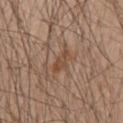Assessment:
Captured during whole-body skin photography for melanoma surveillance; the lesion was not biopsied.
Image and clinical context:
The recorded lesion diameter is about 3 mm. The lesion is on the mid back. A male subject aged approximately 60. An algorithmic analysis of the crop reported a symmetry-axis asymmetry near 0.25. This is a white-light tile. A lesion tile, about 15 mm wide, cut from a 3D total-body photograph.